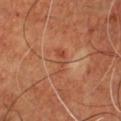Impression: Imaged during a routine full-body skin examination; the lesion was not biopsied and no histopathology is available. Background: The lesion is located on the chest. A region of skin cropped from a whole-body photographic capture, roughly 15 mm wide. The recorded lesion diameter is about 3.5 mm. An algorithmic analysis of the crop reported an eccentricity of roughly 0.95 and a symmetry-axis asymmetry near 0.45. The analysis additionally found roughly 6 lightness units darker than nearby skin and a normalized border contrast of about 5. This is a cross-polarized tile. A male subject aged 43 to 47.